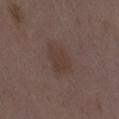A female subject about 35 years old.
A region of skin cropped from a whole-body photographic capture, roughly 15 mm wide.
The lesion is on the right thigh.